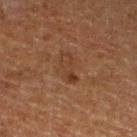follow-up: catalogued during a skin exam; not biopsied | image source: total-body-photography crop, ~15 mm field of view | image-analysis metrics: a lesion color around L≈30 a*≈17 b*≈25 in CIELAB, about 5 CIELAB-L* units darker than the surrounding skin, and a lesion-to-skin contrast of about 5.5 (normalized; higher = more distinct); a border-irregularity rating of about 3/10, a color-variation rating of about 3.5/10, and radial color variation of about 1; an automated nevus-likeness rating near 5 out of 100 and lesion-presence confidence of about 100/100 | subject: male, in their mid- to late 70s | tile lighting: cross-polarized illumination | lesion diameter: ≈3.5 mm | body site: the left lower leg.